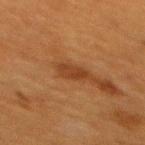Part of a total-body skin-imaging series; this lesion was reviewed on a skin check and was not flagged for biopsy. The subject is a female aged around 40. From the upper back. A 15 mm crop from a total-body photograph taken for skin-cancer surveillance. Approximately 2.5 mm at its widest. Imaged with cross-polarized lighting.